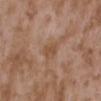Part of a total-body skin-imaging series; this lesion was reviewed on a skin check and was not flagged for biopsy. Captured under white-light illumination. The lesion's longest dimension is about 2.5 mm. A female patient, approximately 35 years of age. A 15 mm close-up extracted from a 3D total-body photography capture. Located on the upper back.